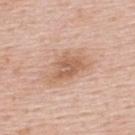{"biopsy_status": "not biopsied; imaged during a skin examination", "patient": {"sex": "male", "age_approx": 55}, "image": {"source": "total-body photography crop", "field_of_view_mm": 15}, "site": "upper back", "lesion_size": {"long_diameter_mm_approx": 5.0}, "automated_metrics": {"area_mm2_approx": 10.0, "shape_asymmetry": 0.35, "cielab_L": 61, "cielab_a": 21, "cielab_b": 31, "vs_skin_darker_L": 10.0, "vs_skin_contrast_norm": 7.0, "border_irregularity_0_10": 4.0, "color_variation_0_10": 3.0}, "lighting": "white-light"}